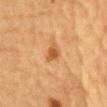The lesion was photographed on a routine skin check and not biopsied; there is no pathology result.
A close-up tile cropped from a whole-body skin photograph, about 15 mm across.
A male subject aged around 85 or older.
From the chest.
The total-body-photography lesion software estimated a lesion area of about 3.5 mm², a shape eccentricity near 0.65, and two-axis asymmetry of about 0.3. It also reported a lesion color around L≈49 a*≈22 b*≈40 in CIELAB, a lesion–skin lightness drop of about 10, and a normalized border contrast of about 7.5. The analysis additionally found border irregularity of about 2.5 on a 0–10 scale, a color-variation rating of about 1.5/10, and radial color variation of about 0.5. It also reported a classifier nevus-likeness of about 85/100 and a lesion-detection confidence of about 100/100.